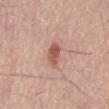Findings:
* follow-up — catalogued during a skin exam; not biopsied
* acquisition — total-body-photography crop, ~15 mm field of view
* subject — male, aged approximately 60
* illumination — white-light illumination
* anatomic site — the mid back